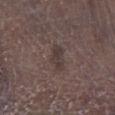biopsy status=imaged on a skin check; not biopsied
lesion size=≈3 mm
patient=male, aged 68–72
anatomic site=the left lower leg
image source=15 mm crop, total-body photography
tile lighting=white-light illumination